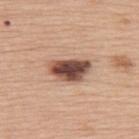Part of a total-body skin-imaging series; this lesion was reviewed on a skin check and was not flagged for biopsy.
A female subject, about 60 years old.
Located on the upper back.
Cropped from a total-body skin-imaging series; the visible field is about 15 mm.